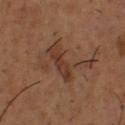Captured during whole-body skin photography for melanoma surveillance; the lesion was not biopsied. The total-body-photography lesion software estimated an average lesion color of about L≈36 a*≈18 b*≈27 (CIELAB), about 7 CIELAB-L* units darker than the surrounding skin, and a lesion-to-skin contrast of about 6.5 (normalized; higher = more distinct). The software also gave a classifier nevus-likeness of about 0/100. The subject is a male aged 53 to 57. About 6.5 mm across. On the upper back. A close-up tile cropped from a whole-body skin photograph, about 15 mm across. The tile uses cross-polarized illumination.